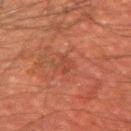<lesion>
  <biopsy_status>not biopsied; imaged during a skin examination</biopsy_status>
  <automated_metrics>
    <lesion_detection_confidence_0_100>100</lesion_detection_confidence_0_100>
  </automated_metrics>
  <site>right upper arm</site>
  <patient>
    <sex>male</sex>
    <age_approx>50</age_approx>
  </patient>
  <lesion_size>
    <long_diameter_mm_approx>2.5</long_diameter_mm_approx>
  </lesion_size>
  <image>
    <source>total-body photography crop</source>
    <field_of_view_mm>15</field_of_view_mm>
  </image>
  <lighting>cross-polarized</lighting>
</lesion>